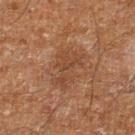Case summary:
• workup — total-body-photography surveillance lesion; no biopsy
• patient — male, about 60 years old
• image — ~15 mm crop, total-body skin-cancer survey
• lighting — cross-polarized
• lesion diameter — about 5 mm
• automated metrics — a lesion area of about 11 mm² and an eccentricity of roughly 0.85
• site — the leg